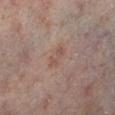| key | value |
|---|---|
| workup | imaged on a skin check; not biopsied |